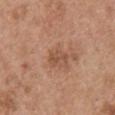The lesion was photographed on a routine skin check and not biopsied; there is no pathology result.
On the chest.
About 3 mm across.
A close-up tile cropped from a whole-body skin photograph, about 15 mm across.
A male subject roughly 50 years of age.